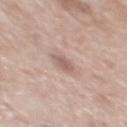follow-up: imaged on a skin check; not biopsied
location: the back
patient: male, roughly 60 years of age
illumination: white-light
image source: total-body-photography crop, ~15 mm field of view